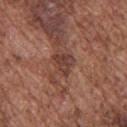Q: Is there a histopathology result?
A: total-body-photography surveillance lesion; no biopsy
Q: What kind of image is this?
A: ~15 mm tile from a whole-body skin photo
Q: Illumination type?
A: white-light
Q: Patient demographics?
A: male, roughly 75 years of age
Q: Where on the body is the lesion?
A: the upper back
Q: What is the lesion's diameter?
A: about 2.5 mm